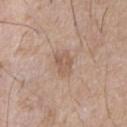Clinical impression: The lesion was photographed on a routine skin check and not biopsied; there is no pathology result. Background: About 3 mm across. Automated tile analysis of the lesion measured a footprint of about 5 mm², an eccentricity of roughly 0.7, and a symmetry-axis asymmetry near 0.3. On the front of the torso. A lesion tile, about 15 mm wide, cut from a 3D total-body photograph. A male subject roughly 65 years of age. This is a white-light tile.